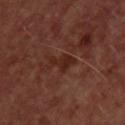biopsy status: catalogued during a skin exam; not biopsied | acquisition: ~15 mm tile from a whole-body skin photo | anatomic site: the upper back | diameter: ≈3.5 mm | subject: female, in their mid- to late 40s.